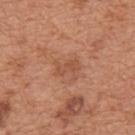Case summary:
• follow-up — imaged on a skin check; not biopsied
• subject — male, roughly 65 years of age
• diameter — ~3 mm (longest diameter)
• anatomic site — the mid back
• image source — total-body-photography crop, ~15 mm field of view
• automated metrics — a lesion color around L≈52 a*≈25 b*≈33 in CIELAB, about 7 CIELAB-L* units darker than the surrounding skin, and a normalized lesion–skin contrast near 5; border irregularity of about 3.5 on a 0–10 scale and a peripheral color-asymmetry measure near 0.5; a nevus-likeness score of about 0/100 and a detector confidence of about 100 out of 100 that the crop contains a lesion